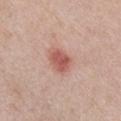The lesion was photographed on a routine skin check and not biopsied; there is no pathology result.
A female subject, aged 48 to 52.
Automated tile analysis of the lesion measured an outline eccentricity of about 0.7 (0 = round, 1 = elongated). The software also gave a lesion color around L≈56 a*≈27 b*≈26 in CIELAB, a lesion–skin lightness drop of about 12, and a normalized border contrast of about 8. The software also gave internal color variation of about 2.5 on a 0–10 scale.
From the chest.
Cropped from a total-body skin-imaging series; the visible field is about 15 mm.
This is a white-light tile.
Longest diameter approximately 3 mm.